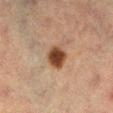The lesion was photographed on a routine skin check and not biopsied; there is no pathology result.
A close-up tile cropped from a whole-body skin photograph, about 15 mm across.
The lesion-visualizer software estimated an automated nevus-likeness rating near 100 out of 100 and a detector confidence of about 100 out of 100 that the crop contains a lesion.
From the left lower leg.
The tile uses cross-polarized illumination.
The lesion's longest dimension is about 3 mm.
A female subject approximately 55 years of age.